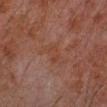{"biopsy_status": "not biopsied; imaged during a skin examination", "patient": {"sex": "male", "age_approx": 30}, "image": {"source": "total-body photography crop", "field_of_view_mm": 15}, "lighting": "cross-polarized", "automated_metrics": {"cielab_L": 34, "cielab_a": 19, "cielab_b": 24, "vs_skin_darker_L": 4.0, "vs_skin_contrast_norm": 5.0, "border_irregularity_0_10": 3.0, "peripheral_color_asymmetry": 1.0}, "site": "right forearm", "lesion_size": {"long_diameter_mm_approx": 3.0}}